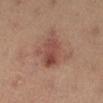The lesion was photographed on a routine skin check and not biopsied; there is no pathology result. The patient is a female roughly 35 years of age. The lesion is located on the right lower leg. A 15 mm close-up tile from a total-body photography series done for melanoma screening.